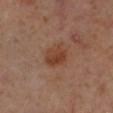Q: Was a biopsy performed?
A: no biopsy performed (imaged during a skin exam)
Q: What is the lesion's diameter?
A: ~3 mm (longest diameter)
Q: Automated lesion metrics?
A: a border-irregularity rating of about 2.5/10, a color-variation rating of about 3.5/10, and radial color variation of about 1.5; an automated nevus-likeness rating near 55 out of 100 and a detector confidence of about 100 out of 100 that the crop contains a lesion
Q: Where on the body is the lesion?
A: the right lower leg
Q: What lighting was used for the tile?
A: cross-polarized
Q: Who is the patient?
A: male, roughly 60 years of age
Q: How was this image acquired?
A: 15 mm crop, total-body photography